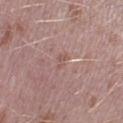workup: total-body-photography surveillance lesion; no biopsy | TBP lesion metrics: a footprint of about 2.5 mm² and a shape-asymmetry score of about 0.45 (0 = symmetric); an average lesion color of about L≈54 a*≈20 b*≈23 (CIELAB), roughly 6 lightness units darker than nearby skin, and a lesion-to-skin contrast of about 4.5 (normalized; higher = more distinct); a lesion-detection confidence of about 65/100 | patient: male, about 40 years old | imaging modality: ~15 mm crop, total-body skin-cancer survey | location: the leg | diameter: about 2.5 mm | illumination: white-light.